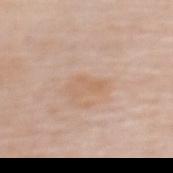{"biopsy_status": "not biopsied; imaged during a skin examination", "patient": {"sex": "female", "age_approx": 50}, "site": "upper back", "image": {"source": "total-body photography crop", "field_of_view_mm": 15}, "automated_metrics": {"eccentricity": 0.9, "shape_asymmetry": 0.45}, "lighting": "white-light", "lesion_size": {"long_diameter_mm_approx": 3.5}}